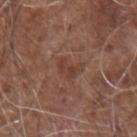This lesion was catalogued during total-body skin photography and was not selected for biopsy.
The patient is a male aged around 75.
The lesion is on the right upper arm.
The recorded lesion diameter is about 3 mm.
The tile uses white-light illumination.
Automated tile analysis of the lesion measured a lesion area of about 4 mm² and an eccentricity of roughly 0.8. The software also gave a border-irregularity index near 5/10, a within-lesion color-variation index near 1.5/10, and peripheral color asymmetry of about 0.5.
A close-up tile cropped from a whole-body skin photograph, about 15 mm across.